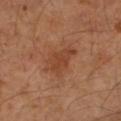Part of a total-body skin-imaging series; this lesion was reviewed on a skin check and was not flagged for biopsy. A female subject aged approximately 60. This image is a 15 mm lesion crop taken from a total-body photograph. The lesion's longest dimension is about 4 mm. Captured under cross-polarized illumination. The lesion is located on the left forearm.